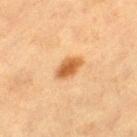Impression:
Imaged during a routine full-body skin examination; the lesion was not biopsied and no histopathology is available.
Clinical summary:
This image is a 15 mm lesion crop taken from a total-body photograph. The lesion is on the left thigh. The total-body-photography lesion software estimated a lesion area of about 5.5 mm² and an outline eccentricity of about 0.75 (0 = round, 1 = elongated). The analysis additionally found a border-irregularity index near 1.5/10, internal color variation of about 3 on a 0–10 scale, and peripheral color asymmetry of about 1. It also reported lesion-presence confidence of about 100/100. Imaged with cross-polarized lighting. The patient is a female about 40 years old.